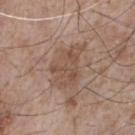Imaged during a routine full-body skin examination; the lesion was not biopsied and no histopathology is available. A 15 mm crop from a total-body photograph taken for skin-cancer surveillance. The lesion is located on the front of the torso. Captured under white-light illumination. The lesion's longest dimension is about 5 mm. The patient is a male approximately 75 years of age. Automated tile analysis of the lesion measured a border-irregularity index near 4.5/10, a within-lesion color-variation index near 3/10, and a peripheral color-asymmetry measure near 1. It also reported a nevus-likeness score of about 0/100 and lesion-presence confidence of about 100/100.